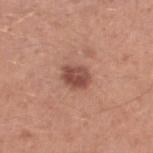On the left lower leg. The subject is a male aged 43–47. A roughly 15 mm field-of-view crop from a total-body skin photograph.